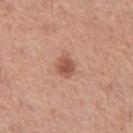Recorded during total-body skin imaging; not selected for excision or biopsy. Captured under white-light illumination. A female subject, aged 53 to 57. From the leg. A roughly 15 mm field-of-view crop from a total-body skin photograph.